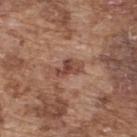The tile uses white-light illumination. The lesion is located on the back. The recorded lesion diameter is about 3.5 mm. The subject is a male roughly 75 years of age. This image is a 15 mm lesion crop taken from a total-body photograph.